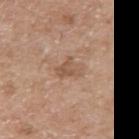Case summary:
- biopsy status: catalogued during a skin exam; not biopsied
- tile lighting: white-light
- size: ~3.5 mm (longest diameter)
- patient: male, in their mid-50s
- body site: the right upper arm
- image source: total-body-photography crop, ~15 mm field of view